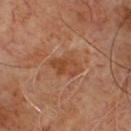The lesion was photographed on a routine skin check and not biopsied; there is no pathology result. The recorded lesion diameter is about 4 mm. A male patient aged approximately 65. An algorithmic analysis of the crop reported an area of roughly 9.5 mm², an outline eccentricity of about 0.75 (0 = round, 1 = elongated), and a symmetry-axis asymmetry near 0.25. It also reported a nevus-likeness score of about 0/100. A 15 mm close-up tile from a total-body photography series done for melanoma screening. This is a cross-polarized tile.